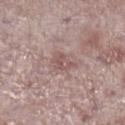| field | value |
|---|---|
| notes | total-body-photography surveillance lesion; no biopsy |
| acquisition | ~15 mm tile from a whole-body skin photo |
| automated metrics | a lesion area of about 4 mm², an eccentricity of roughly 0.75, and a shape-asymmetry score of about 0.35 (0 = symmetric); a lesion color around L≈53 a*≈20 b*≈20 in CIELAB, about 8 CIELAB-L* units darker than the surrounding skin, and a normalized border contrast of about 5.5; a border-irregularity index near 3.5/10 and internal color variation of about 4 on a 0–10 scale; an automated nevus-likeness rating near 0 out of 100 and lesion-presence confidence of about 95/100 |
| size | about 3 mm |
| patient | male, aged approximately 75 |
| site | the leg |